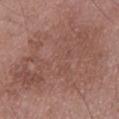Located on the left lower leg. A male subject approximately 50 years of age. The tile uses white-light illumination. Approximately 16 mm at its widest. Automated tile analysis of the lesion measured a footprint of about 85 mm² and an outline eccentricity of about 0.85 (0 = round, 1 = elongated). The software also gave about 7 CIELAB-L* units darker than the surrounding skin. The analysis additionally found a border-irregularity rating of about 6.5/10 and radial color variation of about 1.5. The software also gave a classifier nevus-likeness of about 0/100 and a detector confidence of about 100 out of 100 that the crop contains a lesion. This image is a 15 mm lesion crop taken from a total-body photograph.